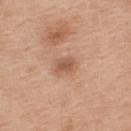follow-up — catalogued during a skin exam; not biopsied
illumination — white-light
size — ~2.5 mm (longest diameter)
acquisition — 15 mm crop, total-body photography
subject — female, aged 38–42
anatomic site — the upper back
automated lesion analysis — roughly 10 lightness units darker than nearby skin and a lesion-to-skin contrast of about 7 (normalized; higher = more distinct); an automated nevus-likeness rating near 30 out of 100